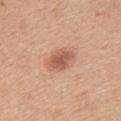Part of a total-body skin-imaging series; this lesion was reviewed on a skin check and was not flagged for biopsy. About 3.5 mm across. A female subject, aged around 45. The total-body-photography lesion software estimated a classifier nevus-likeness of about 95/100 and a detector confidence of about 100 out of 100 that the crop contains a lesion. This is a white-light tile. A close-up tile cropped from a whole-body skin photograph, about 15 mm across. The lesion is on the upper back.